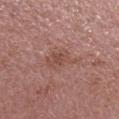| key | value |
|---|---|
| workup | total-body-photography surveillance lesion; no biopsy |
| anatomic site | the left forearm |
| lesion diameter | ≈3.5 mm |
| lighting | white-light illumination |
| image | ~15 mm crop, total-body skin-cancer survey |
| subject | female, aged approximately 50 |